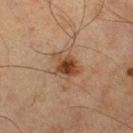Recorded during total-body skin imaging; not selected for excision or biopsy. The subject is a male about 65 years old. Automated tile analysis of the lesion measured a lesion color around L≈36 a*≈17 b*≈28 in CIELAB, a lesion–skin lightness drop of about 10, and a normalized lesion–skin contrast near 9. And it measured a within-lesion color-variation index near 4/10 and a peripheral color-asymmetry measure near 1. And it measured a classifier nevus-likeness of about 95/100. Captured under cross-polarized illumination. The lesion's longest dimension is about 4 mm. Cropped from a whole-body photographic skin survey; the tile spans about 15 mm. From the right lower leg.